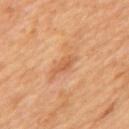No biopsy was performed on this lesion — it was imaged during a full skin examination and was not determined to be concerning. A 15 mm close-up tile from a total-body photography series done for melanoma screening. A male subject, about 65 years old. About 3 mm across. Automated tile analysis of the lesion measured internal color variation of about 1.5 on a 0–10 scale. The software also gave an automated nevus-likeness rating near 0 out of 100 and a lesion-detection confidence of about 100/100. The lesion is located on the back.